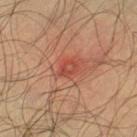biopsy_status: not biopsied; imaged during a skin examination
lighting: cross-polarized
lesion_size:
  long_diameter_mm_approx: 2.5
image:
  source: total-body photography crop
  field_of_view_mm: 15
automated_metrics:
  vs_skin_darker_L: 7.0
  vs_skin_contrast_norm: 6.0
  border_irregularity_0_10: 2.5
  color_variation_0_10: 3.5
  peripheral_color_asymmetry: 1.0
patient:
  sex: male
  age_approx: 35
site: left lower leg